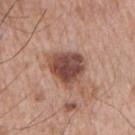follow-up: imaged on a skin check; not biopsied
body site: the right upper arm
automated metrics: an area of roughly 14 mm² and a shape-asymmetry score of about 0.3 (0 = symmetric); a border-irregularity rating of about 3/10, a color-variation rating of about 5/10, and a peripheral color-asymmetry measure near 1.5
illumination: white-light illumination
acquisition: total-body-photography crop, ~15 mm field of view
patient: male, in their mid-60s